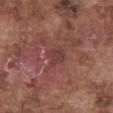– biopsy status: catalogued during a skin exam; not biopsied
– location: the abdomen
– imaging modality: total-body-photography crop, ~15 mm field of view
– patient: male, aged 73 to 77